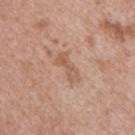Impression: The lesion was photographed on a routine skin check and not biopsied; there is no pathology result. Background: About 4 mm across. On the upper back. A female subject in their 40s. A 15 mm close-up extracted from a 3D total-body photography capture.